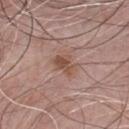<lesion>
  <biopsy_status>not biopsied; imaged during a skin examination</biopsy_status>
  <site>chest</site>
  <lighting>white-light</lighting>
  <patient>
    <sex>male</sex>
    <age_approx>65</age_approx>
  </patient>
  <lesion_size>
    <long_diameter_mm_approx>3.0</long_diameter_mm_approx>
  </lesion_size>
  <image>
    <source>total-body photography crop</source>
    <field_of_view_mm>15</field_of_view_mm>
  </image>
</lesion>